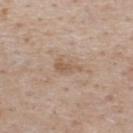This lesion was catalogued during total-body skin photography and was not selected for biopsy. Measured at roughly 3 mm in maximum diameter. A male patient aged approximately 75. Located on the upper back. A region of skin cropped from a whole-body photographic capture, roughly 15 mm wide.